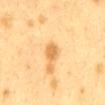* notes — catalogued during a skin exam; not biopsied
* lighting — cross-polarized illumination
* body site — the mid back
* subject — female, approximately 40 years of age
* image source — ~15 mm tile from a whole-body skin photo
* size — about 3 mm